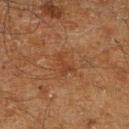Findings:
- follow-up · imaged on a skin check; not biopsied
- lighting · cross-polarized illumination
- image-analysis metrics · a lesion area of about 3.5 mm² and a shape-asymmetry score of about 0.45 (0 = symmetric); about 5 CIELAB-L* units darker than the surrounding skin and a lesion-to-skin contrast of about 5 (normalized; higher = more distinct); border irregularity of about 5.5 on a 0–10 scale, a color-variation rating of about 0/10, and a peripheral color-asymmetry measure near 0; a classifier nevus-likeness of about 0/100 and lesion-presence confidence of about 100/100
- anatomic site · the right lower leg
- patient · male, aged 58–62
- size · ≈3 mm
- image · ~15 mm crop, total-body skin-cancer survey